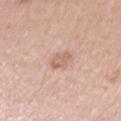notes — no biopsy performed (imaged during a skin exam)
image source — 15 mm crop, total-body photography
TBP lesion metrics — a footprint of about 4 mm²; a mean CIELAB color near L≈63 a*≈19 b*≈27 and a lesion-to-skin contrast of about 6 (normalized; higher = more distinct); a border-irregularity index near 2.5/10, internal color variation of about 2.5 on a 0–10 scale, and a peripheral color-asymmetry measure near 1; a nevus-likeness score of about 0/100 and lesion-presence confidence of about 100/100
lesion size — about 3 mm
subject — female, aged 58–62
location — the left upper arm